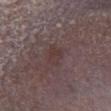{"biopsy_status": "not biopsied; imaged during a skin examination", "lesion_size": {"long_diameter_mm_approx": 2.5}, "site": "right lower leg", "automated_metrics": {"area_mm2_approx": 5.0, "shape_asymmetry": 0.25, "cielab_L": 36, "cielab_a": 16, "cielab_b": 17, "vs_skin_contrast_norm": 5.0, "nevus_likeness_0_100": 0, "lesion_detection_confidence_0_100": 100}, "lighting": "white-light", "image": {"source": "total-body photography crop", "field_of_view_mm": 15}, "patient": {"sex": "male", "age_approx": 65}}